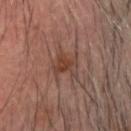{"lesion_size": {"long_diameter_mm_approx": 3.5}, "site": "head or neck", "patient": {"sex": "male", "age_approx": 65}, "lighting": "cross-polarized", "image": {"source": "total-body photography crop", "field_of_view_mm": 15}}